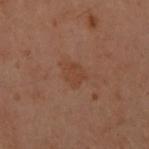notes: catalogued during a skin exam; not biopsied | imaging modality: ~15 mm tile from a whole-body skin photo | site: the front of the torso | lighting: cross-polarized illumination | subject: female, aged 58 to 62 | diameter: about 2.5 mm.